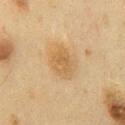Q: Is there a histopathology result?
A: catalogued during a skin exam; not biopsied
Q: Illumination type?
A: cross-polarized illumination
Q: Lesion location?
A: the chest
Q: How large is the lesion?
A: ≈4.5 mm
Q: What are the patient's age and sex?
A: male, aged 58–62
Q: What is the imaging modality?
A: ~15 mm tile from a whole-body skin photo